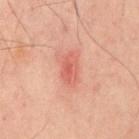| key | value |
|---|---|
| biopsy status | total-body-photography surveillance lesion; no biopsy |
| image-analysis metrics | a classifier nevus-likeness of about 65/100 and a detector confidence of about 100 out of 100 that the crop contains a lesion |
| location | the chest |
| tile lighting | cross-polarized |
| image source | ~15 mm crop, total-body skin-cancer survey |
| lesion size | ≈4 mm |
| subject | male, in their mid- to late 60s |